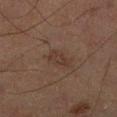workup = no biopsy performed (imaged during a skin exam); image = total-body-photography crop, ~15 mm field of view; TBP lesion metrics = an area of roughly 4 mm², an eccentricity of roughly 0.9, and two-axis asymmetry of about 0.35; subject = male, aged approximately 65; anatomic site = the left lower leg; size = ≈3.5 mm; lighting = cross-polarized illumination.